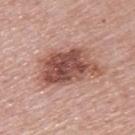Notes:
• biopsy status: no biopsy performed (imaged during a skin exam)
• patient: female, aged 48 to 52
• image: total-body-photography crop, ~15 mm field of view
• anatomic site: the upper back
• diameter: ≈7.5 mm
• automated lesion analysis: a lesion area of about 24 mm², an outline eccentricity of about 0.8 (0 = round, 1 = elongated), and two-axis asymmetry of about 0.3; a lesion color around L≈51 a*≈23 b*≈26 in CIELAB, a lesion–skin lightness drop of about 15, and a lesion-to-skin contrast of about 10 (normalized; higher = more distinct); peripheral color asymmetry of about 2.5; lesion-presence confidence of about 100/100